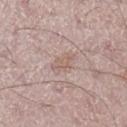Part of a total-body skin-imaging series; this lesion was reviewed on a skin check and was not flagged for biopsy. Imaged with white-light lighting. From the right lower leg. A male patient, about 55 years old. A 15 mm crop from a total-body photograph taken for skin-cancer surveillance.